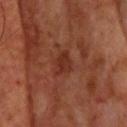This lesion was catalogued during total-body skin photography and was not selected for biopsy.
Cropped from a whole-body photographic skin survey; the tile spans about 15 mm.
A male subject, in their 70s.
The lesion is on the head or neck.
The recorded lesion diameter is about 2.5 mm.
The tile uses cross-polarized illumination.